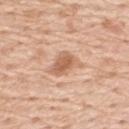biopsy status — catalogued during a skin exam; not biopsied
tile lighting — white-light
automated lesion analysis — a lesion area of about 7 mm² and a shape eccentricity near 0.75; a mean CIELAB color near L≈62 a*≈21 b*≈33, about 11 CIELAB-L* units darker than the surrounding skin, and a lesion-to-skin contrast of about 7 (normalized; higher = more distinct); a classifier nevus-likeness of about 60/100 and a detector confidence of about 100 out of 100 that the crop contains a lesion
image source — 15 mm crop, total-body photography
diameter — ≈4 mm
patient — male, aged 58–62
body site — the back